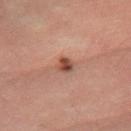{"biopsy_status": "not biopsied; imaged during a skin examination", "lesion_size": {"long_diameter_mm_approx": 2.5}, "automated_metrics": {"border_irregularity_0_10": 2.0, "peripheral_color_asymmetry": 1.5, "nevus_likeness_0_100": 70, "lesion_detection_confidence_0_100": 100}, "lighting": "cross-polarized", "patient": {"sex": "male", "age_approx": 35}, "image": {"source": "total-body photography crop", "field_of_view_mm": 15}, "site": "right forearm"}